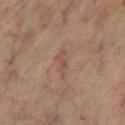workup: total-body-photography surveillance lesion; no biopsy
imaging modality: ~15 mm tile from a whole-body skin photo
anatomic site: the right thigh
subject: female, aged 63 to 67